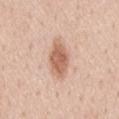The lesion was tiled from a total-body skin photograph and was not biopsied. The subject is a male aged 58 to 62. Cropped from a whole-body photographic skin survey; the tile spans about 15 mm. On the back. An algorithmic analysis of the crop reported a footprint of about 9 mm², a shape eccentricity near 0.9, and two-axis asymmetry of about 0.2. The software also gave a mean CIELAB color near L≈62 a*≈22 b*≈31 and roughly 13 lightness units darker than nearby skin. The software also gave a border-irregularity rating of about 2.5/10, a within-lesion color-variation index near 3/10, and a peripheral color-asymmetry measure near 1. The analysis additionally found an automated nevus-likeness rating near 85 out of 100 and a detector confidence of about 100 out of 100 that the crop contains a lesion. Imaged with white-light lighting.